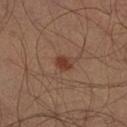Imaged during a routine full-body skin examination; the lesion was not biopsied and no histopathology is available. This image is a 15 mm lesion crop taken from a total-body photograph. The lesion is on the left leg. The patient is a male aged approximately 35.